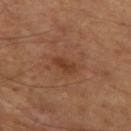| key | value |
|---|---|
| lighting | cross-polarized |
| subject | male, roughly 65 years of age |
| image | total-body-photography crop, ~15 mm field of view |
| diameter | ~3 mm (longest diameter) |